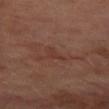{"biopsy_status": "not biopsied; imaged during a skin examination", "patient": {"sex": "male", "age_approx": 70}, "automated_metrics": {"shape_asymmetry": 0.45, "color_variation_0_10": 0.5, "peripheral_color_asymmetry": 0.0, "nevus_likeness_0_100": 0, "lesion_detection_confidence_0_100": 75}, "lighting": "cross-polarized", "lesion_size": {"long_diameter_mm_approx": 3.0}, "site": "left thigh", "image": {"source": "total-body photography crop", "field_of_view_mm": 15}}